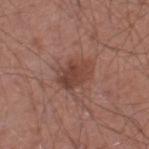The lesion was tiled from a total-body skin photograph and was not biopsied.
About 4 mm across.
A 15 mm close-up extracted from a 3D total-body photography capture.
The lesion-visualizer software estimated an area of roughly 8.5 mm² and an outline eccentricity of about 0.75 (0 = round, 1 = elongated). The analysis additionally found a mean CIELAB color near L≈43 a*≈22 b*≈25, roughly 9 lightness units darker than nearby skin, and a normalized lesion–skin contrast near 7. It also reported a border-irregularity rating of about 2.5/10, a color-variation rating of about 4/10, and a peripheral color-asymmetry measure near 1.5.
A male subject, aged approximately 55.
From the left thigh.